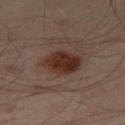{
  "biopsy_status": "not biopsied; imaged during a skin examination",
  "lesion_size": {
    "long_diameter_mm_approx": 4.0
  },
  "site": "leg",
  "image": {
    "source": "total-body photography crop",
    "field_of_view_mm": 15
  },
  "patient": {
    "sex": "male",
    "age_approx": 50
  },
  "automated_metrics": {
    "area_mm2_approx": 12.0,
    "eccentricity": 0.55,
    "shape_asymmetry": 0.15,
    "cielab_L": 24,
    "cielab_a": 15,
    "cielab_b": 19,
    "vs_skin_contrast_norm": 10.5,
    "border_irregularity_0_10": 1.5,
    "color_variation_0_10": 3.0,
    "peripheral_color_asymmetry": 1.0,
    "nevus_likeness_0_100": 95,
    "lesion_detection_confidence_0_100": 100
  }
}